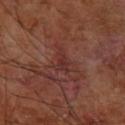Clinical impression:
Part of a total-body skin-imaging series; this lesion was reviewed on a skin check and was not flagged for biopsy.
Image and clinical context:
Cropped from a whole-body photographic skin survey; the tile spans about 15 mm. Imaged with cross-polarized lighting. The recorded lesion diameter is about 2.5 mm. A male subject aged approximately 60. Located on the arm.